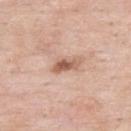Clinical impression: Recorded during total-body skin imaging; not selected for excision or biopsy. Acquisition and patient details: An algorithmic analysis of the crop reported an area of roughly 4.5 mm² and a symmetry-axis asymmetry near 0.35. It also reported a lesion color around L≈59 a*≈21 b*≈30 in CIELAB, roughly 13 lightness units darker than nearby skin, and a lesion-to-skin contrast of about 8.5 (normalized; higher = more distinct). The software also gave a color-variation rating of about 2/10 and peripheral color asymmetry of about 0.5. The subject is a male aged around 55. This is a white-light tile. The lesion is on the upper back. Cropped from a whole-body photographic skin survey; the tile spans about 15 mm.